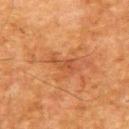A lesion tile, about 15 mm wide, cut from a 3D total-body photograph. The lesion is on the upper back. The recorded lesion diameter is about 3 mm. This is a cross-polarized tile. Automated tile analysis of the lesion measured an average lesion color of about L≈41 a*≈24 b*≈33 (CIELAB) and a lesion-to-skin contrast of about 5.5 (normalized; higher = more distinct). The analysis additionally found border irregularity of about 5.5 on a 0–10 scale, a within-lesion color-variation index near 1/10, and a peripheral color-asymmetry measure near 0.5. A male subject, aged 63–67.